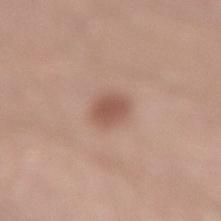Impression:
The lesion was tiled from a total-body skin photograph and was not biopsied.
Image and clinical context:
The total-body-photography lesion software estimated roughly 11 lightness units darker than nearby skin and a lesion-to-skin contrast of about 7.5 (normalized; higher = more distinct). It also reported a border-irregularity index near 2/10, a within-lesion color-variation index near 2/10, and peripheral color asymmetry of about 0.5. This is a white-light tile. A region of skin cropped from a whole-body photographic capture, roughly 15 mm wide. A male patient, about 30 years old. Longest diameter approximately 2.5 mm. The lesion is on the right lower leg.